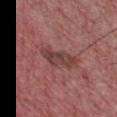Case summary:
* notes · total-body-photography surveillance lesion; no biopsy
* lesion diameter · about 4.5 mm
* automated lesion analysis · a lesion color around L≈41 a*≈23 b*≈22 in CIELAB and a normalized lesion–skin contrast near 7; a within-lesion color-variation index near 3.5/10
* image source · total-body-photography crop, ~15 mm field of view
* subject · male, aged around 65
* anatomic site · the front of the torso